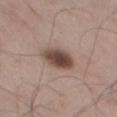workup: catalogued during a skin exam; not biopsied
image: ~15 mm crop, total-body skin-cancer survey
body site: the left thigh
patient: male, about 60 years old
tile lighting: white-light illumination
diameter: ≈4.5 mm